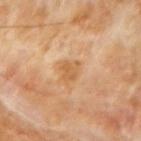Captured during whole-body skin photography for melanoma surveillance; the lesion was not biopsied. On the left forearm. About 3 mm across. The patient is a male aged 68 to 72. This image is a 15 mm lesion crop taken from a total-body photograph.